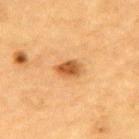Assessment:
Imaged during a routine full-body skin examination; the lesion was not biopsied and no histopathology is available.
Clinical summary:
The patient is a male in their mid-80s or older. An algorithmic analysis of the crop reported a lesion area of about 5.5 mm², an outline eccentricity of about 0.8 (0 = round, 1 = elongated), and two-axis asymmetry of about 0.15. The analysis additionally found a within-lesion color-variation index near 4/10 and radial color variation of about 1.5. The lesion is on the upper back. This is a cross-polarized tile. A lesion tile, about 15 mm wide, cut from a 3D total-body photograph.